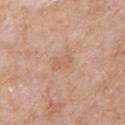Assessment:
Part of a total-body skin-imaging series; this lesion was reviewed on a skin check and was not flagged for biopsy.
Context:
Located on the chest. A close-up tile cropped from a whole-body skin photograph, about 15 mm across. A male subject, aged 58–62.